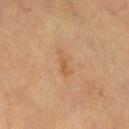Part of a total-body skin-imaging series; this lesion was reviewed on a skin check and was not flagged for biopsy. The patient is aged 58 to 62. From the leg. Imaged with cross-polarized lighting. A 15 mm crop from a total-body photograph taken for skin-cancer surveillance.